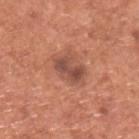biopsy status: total-body-photography surveillance lesion; no biopsy | lesion size: ~4 mm (longest diameter) | imaging modality: total-body-photography crop, ~15 mm field of view | patient: male, approximately 65 years of age | anatomic site: the back | lighting: white-light illumination.